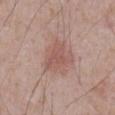{
  "biopsy_status": "not biopsied; imaged during a skin examination",
  "image": {
    "source": "total-body photography crop",
    "field_of_view_mm": 15
  },
  "automated_metrics": {
    "vs_skin_darker_L": 7.0,
    "vs_skin_contrast_norm": 5.5
  },
  "lesion_size": {
    "long_diameter_mm_approx": 4.0
  },
  "site": "abdomen",
  "patient": {
    "sex": "male",
    "age_approx": 70
  },
  "lighting": "white-light"
}